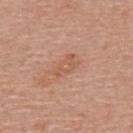Impression:
The lesion was photographed on a routine skin check and not biopsied; there is no pathology result.
Context:
The lesion is located on the mid back. The subject is a male about 60 years old. The total-body-photography lesion software estimated a mean CIELAB color near L≈57 a*≈23 b*≈32, a lesion–skin lightness drop of about 6, and a lesion-to-skin contrast of about 5 (normalized; higher = more distinct). And it measured border irregularity of about 3.5 on a 0–10 scale and internal color variation of about 2 on a 0–10 scale. This image is a 15 mm lesion crop taken from a total-body photograph. Imaged with white-light lighting. Approximately 3.5 mm at its widest.